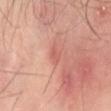Imaged during a routine full-body skin examination; the lesion was not biopsied and no histopathology is available.
On the mid back.
About 2.5 mm across.
A male patient, aged 43 to 47.
A 15 mm close-up extracted from a 3D total-body photography capture.
Captured under cross-polarized illumination.